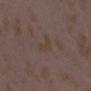{
  "biopsy_status": "not biopsied; imaged during a skin examination",
  "patient": {
    "sex": "female",
    "age_approx": 35
  },
  "lighting": "white-light",
  "image": {
    "source": "total-body photography crop",
    "field_of_view_mm": 15
  },
  "site": "right forearm",
  "lesion_size": {
    "long_diameter_mm_approx": 3.0
  }
}